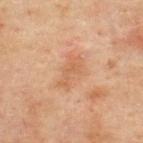Q: Is there a histopathology result?
A: total-body-photography surveillance lesion; no biopsy